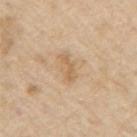  biopsy_status: not biopsied; imaged during a skin examination
  lesion_size:
    long_diameter_mm_approx: 3.0
  site: arm
  patient:
    sex: male
    age_approx: 70
  image:
    source: total-body photography crop
    field_of_view_mm: 15